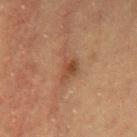| key | value |
|---|---|
| biopsy status | catalogued during a skin exam; not biopsied |
| illumination | cross-polarized |
| image source | 15 mm crop, total-body photography |
| site | the mid back |
| diameter | ~4 mm (longest diameter) |
| subject | female, aged approximately 70 |